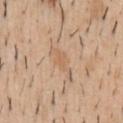This lesion was catalogued during total-body skin photography and was not selected for biopsy.
A male patient in their 40s.
From the chest.
A 15 mm crop from a total-body photograph taken for skin-cancer surveillance.